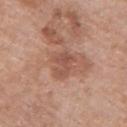The lesion was tiled from a total-body skin photograph and was not biopsied.
From the upper back.
The subject is a male roughly 70 years of age.
Approximately 3 mm at its widest.
A close-up tile cropped from a whole-body skin photograph, about 15 mm across.